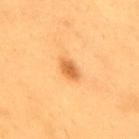notes: total-body-photography surveillance lesion; no biopsy | anatomic site: the upper back | tile lighting: cross-polarized | acquisition: total-body-photography crop, ~15 mm field of view | subject: male, about 60 years old.